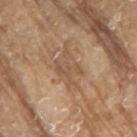Notes:
– workup · no biopsy performed (imaged during a skin exam)
– image-analysis metrics · an eccentricity of roughly 0.85 and two-axis asymmetry of about 0.4; a mean CIELAB color near L≈54 a*≈17 b*≈32 and a lesion-to-skin contrast of about 5.5 (normalized; higher = more distinct); a nevus-likeness score of about 0/100 and a detector confidence of about 55 out of 100 that the crop contains a lesion
– body site · the arm
– lighting · white-light illumination
– image source · total-body-photography crop, ~15 mm field of view
– subject · male, aged approximately 80